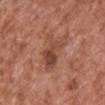Impression:
This lesion was catalogued during total-body skin photography and was not selected for biopsy.